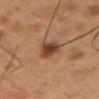Assessment: The lesion was tiled from a total-body skin photograph and was not biopsied. Context: A male subject aged 48–52. From the upper back. A 15 mm close-up tile from a total-body photography series done for melanoma screening.